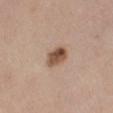<record>
  <lesion_size>
    <long_diameter_mm_approx>3.0</long_diameter_mm_approx>
  </lesion_size>
  <patient>
    <sex>female</sex>
    <age_approx>60</age_approx>
  </patient>
  <image>
    <source>total-body photography crop</source>
    <field_of_view_mm>15</field_of_view_mm>
  </image>
  <site>leg</site>
</record>